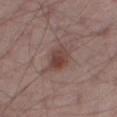Assessment:
Recorded during total-body skin imaging; not selected for excision or biopsy.
Acquisition and patient details:
This image is a 15 mm lesion crop taken from a total-body photograph. A male patient, about 65 years old. On the right thigh.